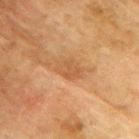Q: Was a biopsy performed?
A: no biopsy performed (imaged during a skin exam)
Q: What kind of image is this?
A: total-body-photography crop, ~15 mm field of view
Q: What are the patient's age and sex?
A: male, roughly 75 years of age
Q: Lesion location?
A: the upper back